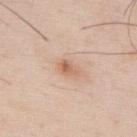biopsy_status: not biopsied; imaged during a skin examination
automated_metrics:
  area_mm2_approx: 3.0
  eccentricity: 0.8
  shape_asymmetry: 0.35
  cielab_L: 63
  cielab_a: 21
  cielab_b: 32
  vs_skin_darker_L: 10.0
  vs_skin_contrast_norm: 7.0
lesion_size:
  long_diameter_mm_approx: 2.5
image:
  source: total-body photography crop
  field_of_view_mm: 15
patient:
  sex: male
  age_approx: 55
site: back
lighting: white-light